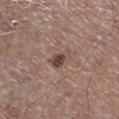The lesion was tiled from a total-body skin photograph and was not biopsied. A 15 mm close-up extracted from a 3D total-body photography capture. The total-body-photography lesion software estimated a lesion area of about 3.5 mm², a shape eccentricity near 0.8, and a shape-asymmetry score of about 0.35 (0 = symmetric). The analysis additionally found an average lesion color of about L≈44 a*≈18 b*≈21 (CIELAB), about 12 CIELAB-L* units darker than the surrounding skin, and a lesion-to-skin contrast of about 9 (normalized; higher = more distinct). A male subject aged approximately 70. Approximately 2.5 mm at its widest. On the right lower leg.